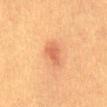biopsy status — no biopsy performed (imaged during a skin exam); illumination — cross-polarized illumination; imaging modality — 15 mm crop, total-body photography; lesion diameter — ≈3 mm; patient — female, aged approximately 40; site — the abdomen.